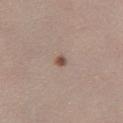<record>
  <biopsy_status>not biopsied; imaged during a skin examination</biopsy_status>
  <patient>
    <sex>female</sex>
    <age_approx>20</age_approx>
  </patient>
  <image>
    <source>total-body photography crop</source>
    <field_of_view_mm>15</field_of_view_mm>
  </image>
  <site>left lower leg</site>
  <lesion_size>
    <long_diameter_mm_approx>1.5</long_diameter_mm_approx>
  </lesion_size>
  <lighting>white-light</lighting>
</record>